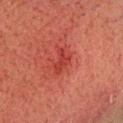Part of a total-body skin-imaging series; this lesion was reviewed on a skin check and was not flagged for biopsy. The lesion's longest dimension is about 3 mm. The lesion-visualizer software estimated an area of roughly 4.5 mm² and two-axis asymmetry of about 0.4. The analysis additionally found roughly 7 lightness units darker than nearby skin and a normalized lesion–skin contrast near 6. A male subject aged approximately 50. On the head or neck. Cropped from a whole-body photographic skin survey; the tile spans about 15 mm.